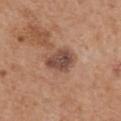Imaged during a routine full-body skin examination; the lesion was not biopsied and no histopathology is available. Cropped from a total-body skin-imaging series; the visible field is about 15 mm. A male subject, aged 68 to 72. The total-body-photography lesion software estimated a border-irregularity index near 2/10. The lesion's longest dimension is about 3.5 mm. Located on the mid back. Captured under white-light illumination.